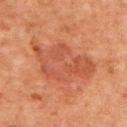Q: Was this lesion biopsied?
A: no biopsy performed (imaged during a skin exam)
Q: Illumination type?
A: cross-polarized illumination
Q: What kind of image is this?
A: total-body-photography crop, ~15 mm field of view
Q: Lesion location?
A: the upper back
Q: What did automated image analysis measure?
A: a lesion area of about 25 mm², an eccentricity of roughly 0.85, and two-axis asymmetry of about 0.35; a border-irregularity index near 4.5/10, internal color variation of about 3.5 on a 0–10 scale, and a peripheral color-asymmetry measure near 1; a nevus-likeness score of about 10/100 and a lesion-detection confidence of about 100/100
Q: Patient demographics?
A: male, aged 78–82
Q: Lesion size?
A: ≈8.5 mm